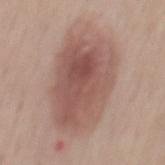Impression: No biopsy was performed on this lesion — it was imaged during a full skin examination and was not determined to be concerning. Acquisition and patient details: The subject is a male roughly 55 years of age. A 15 mm crop from a total-body photograph taken for skin-cancer surveillance. Located on the mid back.